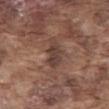Assessment:
Part of a total-body skin-imaging series; this lesion was reviewed on a skin check and was not flagged for biopsy.
Clinical summary:
On the abdomen. The subject is a male in their mid- to late 70s. Automated tile analysis of the lesion measured a shape-asymmetry score of about 0.25 (0 = symmetric). It also reported a color-variation rating of about 2.5/10 and radial color variation of about 1. Imaged with white-light lighting. Cropped from a whole-body photographic skin survey; the tile spans about 15 mm. Longest diameter approximately 4 mm.